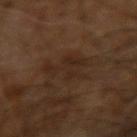notes: total-body-photography surveillance lesion; no biopsy
site: the right arm
lighting: cross-polarized illumination
lesion size: ≈5 mm
automated lesion analysis: an automated nevus-likeness rating near 0 out of 100 and a detector confidence of about 100 out of 100 that the crop contains a lesion
image: total-body-photography crop, ~15 mm field of view
patient: male, in their 60s